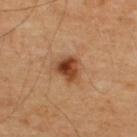A male patient aged around 50. On the upper back. A lesion tile, about 15 mm wide, cut from a 3D total-body photograph. This is a cross-polarized tile.